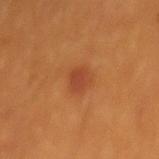The lesion is located on the back. Measured at roughly 2.5 mm in maximum diameter. A male subject aged approximately 60. The tile uses cross-polarized illumination. The total-body-photography lesion software estimated a mean CIELAB color near L≈41 a*≈28 b*≈35, about 7 CIELAB-L* units darker than the surrounding skin, and a normalized lesion–skin contrast near 6.5. The analysis additionally found border irregularity of about 2 on a 0–10 scale. A 15 mm close-up extracted from a 3D total-body photography capture.